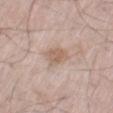A 15 mm close-up extracted from a 3D total-body photography capture.
Approximately 3 mm at its widest.
This is a white-light tile.
On the right thigh.
A male patient aged 68 to 72.
An algorithmic analysis of the crop reported a lesion area of about 5.5 mm², a shape eccentricity near 0.55, and a shape-asymmetry score of about 0.2 (0 = symmetric). The software also gave lesion-presence confidence of about 100/100.